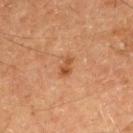Assessment: No biopsy was performed on this lesion — it was imaged during a full skin examination and was not determined to be concerning. Background: Cropped from a whole-body photographic skin survey; the tile spans about 15 mm. The tile uses cross-polarized illumination. Longest diameter approximately 2.5 mm. A male subject, roughly 60 years of age.